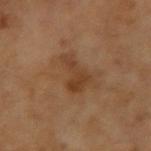This lesion was catalogued during total-body skin photography and was not selected for biopsy.
Longest diameter approximately 4 mm.
The subject is a male aged 63–67.
The lesion is on the left arm.
A close-up tile cropped from a whole-body skin photograph, about 15 mm across.